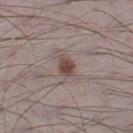notes — total-body-photography surveillance lesion; no biopsy | location — the right lower leg | patient — male, aged approximately 70 | imaging modality — total-body-photography crop, ~15 mm field of view | illumination — white-light illumination.